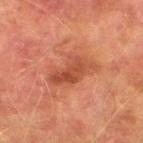{"biopsy_status": "not biopsied; imaged during a skin examination", "lighting": "cross-polarized", "image": {"source": "total-body photography crop", "field_of_view_mm": 15}, "patient": {"sex": "male", "age_approx": 75}, "site": "left lower leg", "lesion_size": {"long_diameter_mm_approx": 5.5}, "automated_metrics": {"border_irregularity_0_10": 4.5, "color_variation_0_10": 4.0, "peripheral_color_asymmetry": 1.5}}